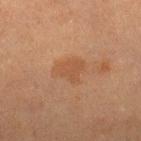The lesion is on the right lower leg.
Imaged with cross-polarized lighting.
Cropped from a total-body skin-imaging series; the visible field is about 15 mm.
Longest diameter approximately 3 mm.
A female subject approximately 55 years of age.